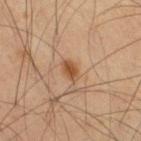Case summary:
- size — ≈2.5 mm
- subject — male, roughly 60 years of age
- tile lighting — cross-polarized illumination
- anatomic site — the left thigh
- image source — total-body-photography crop, ~15 mm field of view